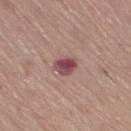follow-up: total-body-photography surveillance lesion; no biopsy
location: the right thigh
patient: female, about 65 years old
image source: total-body-photography crop, ~15 mm field of view